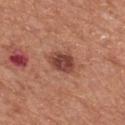biopsy status: no biopsy performed (imaged during a skin exam)
tile lighting: white-light illumination
patient: male, aged around 65
body site: the mid back
image: 15 mm crop, total-body photography
size: ≈3.5 mm
automated metrics: a lesion color around L≈44 a*≈25 b*≈27 in CIELAB and roughly 13 lightness units darker than nearby skin; an automated nevus-likeness rating near 80 out of 100